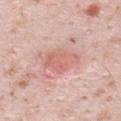Captured during whole-body skin photography for melanoma surveillance; the lesion was not biopsied. This is a white-light tile. The lesion is on the back. An algorithmic analysis of the crop reported a lesion area of about 9.5 mm², an eccentricity of roughly 0.7, and a symmetry-axis asymmetry near 0.25. And it measured about 8 CIELAB-L* units darker than the surrounding skin and a normalized border contrast of about 5.5. The analysis additionally found a border-irregularity rating of about 2.5/10. And it measured an automated nevus-likeness rating near 100 out of 100. A male subject, in their mid-30s. Cropped from a total-body skin-imaging series; the visible field is about 15 mm.